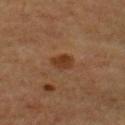{
  "patient": {
    "sex": "male",
    "age_approx": 55
  },
  "image": {
    "source": "total-body photography crop",
    "field_of_view_mm": 15
  },
  "lighting": "cross-polarized",
  "lesion_size": {
    "long_diameter_mm_approx": 2.5
  },
  "site": "chest"
}